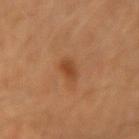{
  "biopsy_status": "not biopsied; imaged during a skin examination",
  "lighting": "cross-polarized",
  "patient": {
    "sex": "male",
    "age_approx": 40
  },
  "site": "left forearm",
  "automated_metrics": {
    "cielab_L": 45,
    "cielab_a": 25,
    "cielab_b": 37,
    "vs_skin_darker_L": 9.0
  },
  "image": {
    "source": "total-body photography crop",
    "field_of_view_mm": 15
  },
  "lesion_size": {
    "long_diameter_mm_approx": 2.5
  }
}